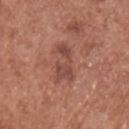Impression: Recorded during total-body skin imaging; not selected for excision or biopsy. Acquisition and patient details: The lesion-visualizer software estimated a mean CIELAB color near L≈47 a*≈24 b*≈27. The tile uses white-light illumination. A 15 mm crop from a total-body photograph taken for skin-cancer surveillance. On the chest. The patient is a male aged 73–77. The lesion's longest dimension is about 4.5 mm.